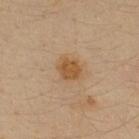automated metrics: a lesion area of about 6 mm², a shape eccentricity near 0.5, and a shape-asymmetry score of about 0.15 (0 = symmetric); a mean CIELAB color near L≈46 a*≈16 b*≈33, about 8 CIELAB-L* units darker than the surrounding skin, and a normalized lesion–skin contrast near 8; border irregularity of about 1.5 on a 0–10 scale, a color-variation rating of about 1.5/10, and radial color variation of about 0.5; a classifier nevus-likeness of about 90/100 and a detector confidence of about 100 out of 100 that the crop contains a lesion | location: the upper back | tile lighting: cross-polarized illumination | patient: male, approximately 35 years of age | imaging modality: ~15 mm tile from a whole-body skin photo | lesion diameter: ~3 mm (longest diameter).